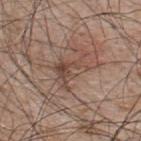A close-up tile cropped from a whole-body skin photograph, about 15 mm across. The lesion's longest dimension is about 5 mm. Imaged with white-light lighting. The patient is a male in their 50s. The lesion is on the back.